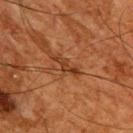Notes:
– biopsy status: catalogued during a skin exam; not biopsied
– body site: the back
– lighting: cross-polarized illumination
– acquisition: 15 mm crop, total-body photography
– lesion size: ≈3.5 mm
– subject: male, aged 63 to 67
– automated metrics: a lesion area of about 3.5 mm² and an eccentricity of roughly 0.95; a lesion color around L≈31 a*≈21 b*≈30 in CIELAB, roughly 8 lightness units darker than nearby skin, and a normalized lesion–skin contrast near 8; a border-irregularity rating of about 6.5/10 and a peripheral color-asymmetry measure near 0